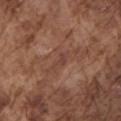Cropped from a total-body skin-imaging series; the visible field is about 15 mm. The recorded lesion diameter is about 2.5 mm. The subject is a male in their mid-70s. Imaged with white-light lighting. On the left upper arm.